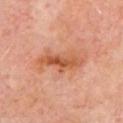About 6 mm across. The subject is aged 63–67. A 15 mm crop from a total-body photograph taken for skin-cancer surveillance. The lesion is on the chest. The tile uses cross-polarized illumination.